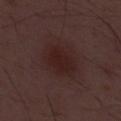Recorded during total-body skin imaging; not selected for excision or biopsy.
A male subject roughly 50 years of age.
Measured at roughly 6 mm in maximum diameter.
Imaged with white-light lighting.
Cropped from a total-body skin-imaging series; the visible field is about 15 mm.